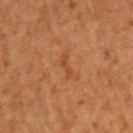Clinical impression: The lesion was tiled from a total-body skin photograph and was not biopsied. Acquisition and patient details: A 15 mm crop from a total-body photograph taken for skin-cancer surveillance. The tile uses cross-polarized illumination. The lesion is located on the upper back. Longest diameter approximately 2.5 mm. The patient is a male roughly 50 years of age.